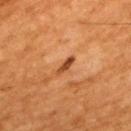Case summary:
* follow-up — total-body-photography surveillance lesion; no biopsy
* patient — male, aged approximately 60
* anatomic site — the upper back
* acquisition — ~15 mm tile from a whole-body skin photo